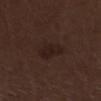follow-up: imaged on a skin check; not biopsied | patient: male, aged 68 to 72 | image: ~15 mm tile from a whole-body skin photo | illumination: white-light illumination | anatomic site: the left lower leg | size: about 3.5 mm.